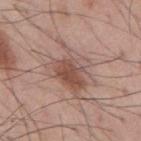Q: What are the patient's age and sex?
A: male, aged approximately 55
Q: What is the anatomic site?
A: the mid back
Q: How was this image acquired?
A: total-body-photography crop, ~15 mm field of view
Q: Lesion size?
A: about 6 mm
Q: What did automated image analysis measure?
A: a shape eccentricity near 0.65 and two-axis asymmetry of about 0.35; a mean CIELAB color near L≈52 a*≈19 b*≈25, roughly 10 lightness units darker than nearby skin, and a lesion-to-skin contrast of about 7 (normalized; higher = more distinct); a nevus-likeness score of about 80/100
Q: Illumination type?
A: white-light illumination